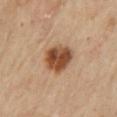<case>
  <biopsy_status>not biopsied; imaged during a skin examination</biopsy_status>
  <patient>
    <sex>female</sex>
    <age_approx>60</age_approx>
  </patient>
  <lesion_size>
    <long_diameter_mm_approx>4.0</long_diameter_mm_approx>
  </lesion_size>
  <site>mid back</site>
  <automated_metrics>
    <nevus_likeness_0_100>100</nevus_likeness_0_100>
    <lesion_detection_confidence_0_100>100</lesion_detection_confidence_0_100>
  </automated_metrics>
  <lighting>cross-polarized</lighting>
  <image>
    <source>total-body photography crop</source>
    <field_of_view_mm>15</field_of_view_mm>
  </image>
</case>